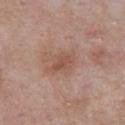Assessment: Recorded during total-body skin imaging; not selected for excision or biopsy. Clinical summary: A 15 mm close-up tile from a total-body photography series done for melanoma screening. The patient is a male in their mid- to late 50s. Approximately 3 mm at its widest. The lesion is located on the chest.